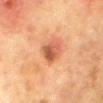biopsy status: no biopsy performed (imaged during a skin exam)
lighting: cross-polarized
acquisition: 15 mm crop, total-body photography
size: ≈3.5 mm
subject: female, about 50 years old
automated lesion analysis: an area of roughly 7 mm², a shape eccentricity near 0.7, and two-axis asymmetry of about 0.25; a border-irregularity index near 2.5/10, internal color variation of about 6.5 on a 0–10 scale, and radial color variation of about 2.5
anatomic site: the chest